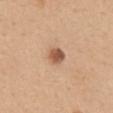Assessment:
This lesion was catalogued during total-body skin photography and was not selected for biopsy.
Background:
The lesion is located on the mid back. A 15 mm crop from a total-body photograph taken for skin-cancer surveillance. Imaged with white-light lighting. The lesion's longest dimension is about 2.5 mm. A male patient, approximately 40 years of age.